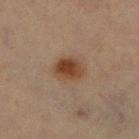Notes:
* notes — imaged on a skin check; not biopsied
* patient — female, about 70 years old
* image — ~15 mm tile from a whole-body skin photo
* anatomic site — the right lower leg
* automated metrics — an area of roughly 8.5 mm², a shape eccentricity near 0.65, and two-axis asymmetry of about 0.15; a lesion color around L≈37 a*≈17 b*≈27 in CIELAB and roughly 9 lightness units darker than nearby skin
* size — ~3.5 mm (longest diameter)